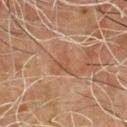notes: no biopsy performed (imaged during a skin exam)
image: 15 mm crop, total-body photography
lesion size: ~2.5 mm (longest diameter)
TBP lesion metrics: a mean CIELAB color near L≈50 a*≈22 b*≈31, a lesion–skin lightness drop of about 6, and a normalized lesion–skin contrast near 5; a border-irregularity index near 5/10, internal color variation of about 1.5 on a 0–10 scale, and peripheral color asymmetry of about 0.5
patient: male, aged approximately 65
tile lighting: cross-polarized illumination
anatomic site: the chest